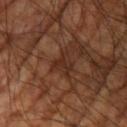Imaged during a routine full-body skin examination; the lesion was not biopsied and no histopathology is available.
This image is a 15 mm lesion crop taken from a total-body photograph.
The lesion is located on the right upper arm.
A male patient approximately 60 years of age.